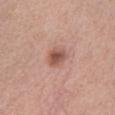Imaged during a routine full-body skin examination; the lesion was not biopsied and no histopathology is available. This image is a 15 mm lesion crop taken from a total-body photograph. On the chest. A female patient aged 38 to 42.